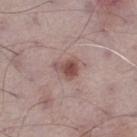Captured during whole-body skin photography for melanoma surveillance; the lesion was not biopsied. A 15 mm close-up tile from a total-body photography series done for melanoma screening. The lesion is on the right thigh. About 3 mm across. A male patient aged approximately 70. An algorithmic analysis of the crop reported a lesion area of about 5.5 mm² and two-axis asymmetry of about 0.4. And it measured a mean CIELAB color near L≈50 a*≈20 b*≈22. And it measured lesion-presence confidence of about 100/100.